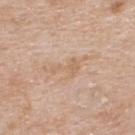A region of skin cropped from a whole-body photographic capture, roughly 15 mm wide. The lesion is located on the upper back. The subject is a male in their mid- to late 60s. Automated tile analysis of the lesion measured an area of roughly 4.5 mm², a shape eccentricity near 0.85, and two-axis asymmetry of about 0.6. It also reported roughly 6 lightness units darker than nearby skin and a normalized border contrast of about 5.